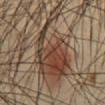workup: imaged on a skin check; not biopsied
site: the abdomen
lesion size: ≈11 mm
image-analysis metrics: an outline eccentricity of about 0.9 (0 = round, 1 = elongated) and two-axis asymmetry of about 0.55; a border-irregularity rating of about 9/10, internal color variation of about 7 on a 0–10 scale, and a peripheral color-asymmetry measure near 2; an automated nevus-likeness rating near 0 out of 100 and a lesion-detection confidence of about 5/100
subject: male, aged around 65
imaging modality: 15 mm crop, total-body photography